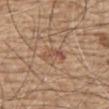Imaged during a routine full-body skin examination; the lesion was not biopsied and no histopathology is available. Automated tile analysis of the lesion measured an average lesion color of about L≈51 a*≈21 b*≈29 (CIELAB), a lesion–skin lightness drop of about 8, and a normalized lesion–skin contrast near 6. And it measured an automated nevus-likeness rating near 0 out of 100 and a detector confidence of about 100 out of 100 that the crop contains a lesion. The lesion is located on the abdomen. A 15 mm crop from a total-body photograph taken for skin-cancer surveillance. A male subject, in their 70s. The tile uses white-light illumination. Longest diameter approximately 3.5 mm.